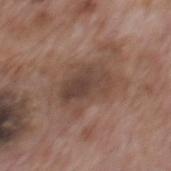Part of a total-body skin-imaging series; this lesion was reviewed on a skin check and was not flagged for biopsy. This image is a 15 mm lesion crop taken from a total-body photograph. A male subject approximately 70 years of age. Located on the mid back. Captured under white-light illumination.